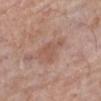Clinical impression: Recorded during total-body skin imaging; not selected for excision or biopsy. Image and clinical context: Longest diameter approximately 3.5 mm. Imaged with white-light lighting. Located on the left lower leg. The patient is a female about 70 years old. A 15 mm close-up extracted from a 3D total-body photography capture.